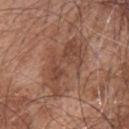Impression:
No biopsy was performed on this lesion — it was imaged during a full skin examination and was not determined to be concerning.
Clinical summary:
From the upper back. A male patient, roughly 45 years of age. A roughly 15 mm field-of-view crop from a total-body skin photograph.